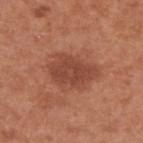biopsy_status: not biopsied; imaged during a skin examination
image:
  source: total-body photography crop
  field_of_view_mm: 15
site: upper back
lighting: white-light
patient:
  sex: male
  age_approx: 55